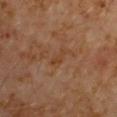<record>
<lighting>cross-polarized</lighting>
<patient>
  <sex>male</sex>
  <age_approx>60</age_approx>
</patient>
<site>right upper arm</site>
<image>
  <source>total-body photography crop</source>
  <field_of_view_mm>15</field_of_view_mm>
</image>
<automated_metrics>
  <area_mm2_approx>3.0</area_mm2_approx>
  <eccentricity>0.9</eccentricity>
  <shape_asymmetry>0.35</shape_asymmetry>
  <cielab_L>38</cielab_L>
  <cielab_a>19</cielab_a>
  <cielab_b>31</cielab_b>
  <vs_skin_darker_L>4.0</vs_skin_darker_L>
</automated_metrics>
<lesion_size>
  <long_diameter_mm_approx>3.0</long_diameter_mm_approx>
</lesion_size>
</record>